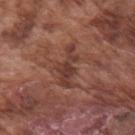notes: imaged on a skin check; not biopsied | tile lighting: white-light | lesion size: about 5 mm | automated lesion analysis: a footprint of about 7.5 mm² and two-axis asymmetry of about 0.45; a border-irregularity rating of about 6.5/10, internal color variation of about 2.5 on a 0–10 scale, and radial color variation of about 0.5 | imaging modality: 15 mm crop, total-body photography | subject: male, about 75 years old | body site: the right upper arm.